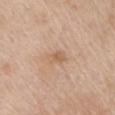follow-up: imaged on a skin check; not biopsied
acquisition: 15 mm crop, total-body photography
anatomic site: the chest
subject: male, about 65 years old